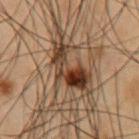follow-up: imaged on a skin check; not biopsied
body site: the chest
acquisition: 15 mm crop, total-body photography
patient: male, in their mid-50s
lesion size: ≈6 mm
illumination: cross-polarized
image-analysis metrics: border irregularity of about 6 on a 0–10 scale and a within-lesion color-variation index near 10/10